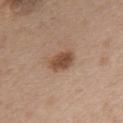Q: Was a biopsy performed?
A: no biopsy performed (imaged during a skin exam)
Q: What lighting was used for the tile?
A: white-light illumination
Q: Automated lesion metrics?
A: an area of roughly 7 mm², a shape eccentricity near 0.75, and two-axis asymmetry of about 0.25; an automated nevus-likeness rating near 95 out of 100
Q: Who is the patient?
A: male, approximately 50 years of age
Q: Lesion size?
A: about 3.5 mm
Q: What kind of image is this?
A: 15 mm crop, total-body photography
Q: Lesion location?
A: the front of the torso